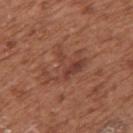<case>
<biopsy_status>not biopsied; imaged during a skin examination</biopsy_status>
<image>
  <source>total-body photography crop</source>
  <field_of_view_mm>15</field_of_view_mm>
</image>
<lighting>white-light</lighting>
<site>upper back</site>
<lesion_size>
  <long_diameter_mm_approx>5.5</long_diameter_mm_approx>
</lesion_size>
<patient>
  <sex>female</sex>
  <age_approx>75</age_approx>
</patient>
</case>